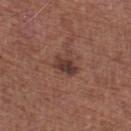{
  "biopsy_status": "not biopsied; imaged during a skin examination",
  "patient": {
    "sex": "male",
    "age_approx": 75
  },
  "image": {
    "source": "total-body photography crop",
    "field_of_view_mm": 15
  },
  "site": "left lower leg"
}